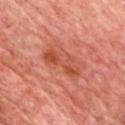Part of a total-body skin-imaging series; this lesion was reviewed on a skin check and was not flagged for biopsy. Approximately 5 mm at its widest. A male subject aged approximately 65. On the chest. The lesion-visualizer software estimated a footprint of about 8.5 mm², a shape eccentricity near 0.95, and a symmetry-axis asymmetry near 0.3. It also reported a within-lesion color-variation index near 5/10 and peripheral color asymmetry of about 1.5. Cropped from a whole-body photographic skin survey; the tile spans about 15 mm. Captured under cross-polarized illumination.